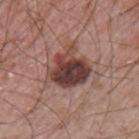Captured during whole-body skin photography for melanoma surveillance; the lesion was not biopsied. Located on the left upper arm. A 15 mm close-up tile from a total-body photography series done for melanoma screening. An algorithmic analysis of the crop reported a shape eccentricity near 0.3 and a shape-asymmetry score of about 0.35 (0 = symmetric). It also reported an average lesion color of about L≈41 a*≈21 b*≈21 (CIELAB) and a lesion-to-skin contrast of about 11.5 (normalized; higher = more distinct). The software also gave border irregularity of about 4 on a 0–10 scale, a within-lesion color-variation index near 8.5/10, and peripheral color asymmetry of about 2.5. Approximately 5 mm at its widest. The subject is a male about 65 years old. Captured under white-light illumination.